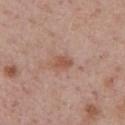biopsy status: total-body-photography surveillance lesion; no biopsy | location: the chest | acquisition: ~15 mm crop, total-body skin-cancer survey | patient: male, aged around 75 | tile lighting: white-light | lesion diameter: about 2.5 mm.